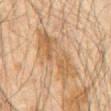Impression: Captured during whole-body skin photography for melanoma surveillance; the lesion was not biopsied. Image and clinical context: The lesion is on the abdomen. Captured under cross-polarized illumination. Cropped from a whole-body photographic skin survey; the tile spans about 15 mm. A male subject in their 60s.